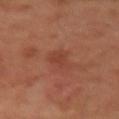Notes:
• notes · no biopsy performed (imaged during a skin exam)
• size · ≈3.5 mm
• image source · 15 mm crop, total-body photography
• patient · female, in their mid- to late 50s
• site · the right upper arm